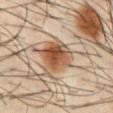follow-up = total-body-photography surveillance lesion; no biopsy
site = the abdomen
subject = male, aged around 40
acquisition = total-body-photography crop, ~15 mm field of view
lesion diameter = ≈4.5 mm
illumination = cross-polarized illumination
TBP lesion metrics = a lesion color around L≈52 a*≈20 b*≈33 in CIELAB and a lesion–skin lightness drop of about 14; border irregularity of about 2.5 on a 0–10 scale, internal color variation of about 8 on a 0–10 scale, and a peripheral color-asymmetry measure near 2.5; a nevus-likeness score of about 100/100